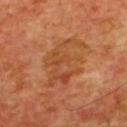No biopsy was performed on this lesion — it was imaged during a full skin examination and was not determined to be concerning. About 7 mm across. A 15 mm crop from a total-body photograph taken for skin-cancer surveillance. This is a cross-polarized tile. The subject is a male aged 63 to 67. The lesion is on the upper back.